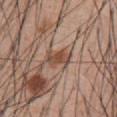Q: What are the patient's age and sex?
A: male, approximately 55 years of age
Q: How large is the lesion?
A: ≈3 mm
Q: Where on the body is the lesion?
A: the front of the torso
Q: Illumination type?
A: white-light
Q: What kind of image is this?
A: ~15 mm crop, total-body skin-cancer survey